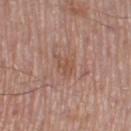| field | value |
|---|---|
| biopsy status | catalogued during a skin exam; not biopsied |
| body site | the right thigh |
| diameter | ~2.5 mm (longest diameter) |
| lighting | white-light |
| image-analysis metrics | a mean CIELAB color near L≈52 a*≈20 b*≈28, a lesion–skin lightness drop of about 6, and a normalized border contrast of about 5; a border-irregularity index near 3.5/10 and internal color variation of about 1.5 on a 0–10 scale; a classifier nevus-likeness of about 0/100 and a lesion-detection confidence of about 100/100 |
| subject | male, in their mid- to late 50s |
| acquisition | 15 mm crop, total-body photography |